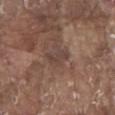Part of a total-body skin-imaging series; this lesion was reviewed on a skin check and was not flagged for biopsy.
Captured under white-light illumination.
A male patient about 80 years old.
From the chest.
Longest diameter approximately 3 mm.
The lesion-visualizer software estimated an eccentricity of roughly 0.8 and two-axis asymmetry of about 0.45. The analysis additionally found a mean CIELAB color near L≈42 a*≈16 b*≈22, about 7 CIELAB-L* units darker than the surrounding skin, and a normalized border contrast of about 5.5. It also reported border irregularity of about 4 on a 0–10 scale, a color-variation rating of about 2/10, and peripheral color asymmetry of about 0.5.
Cropped from a total-body skin-imaging series; the visible field is about 15 mm.